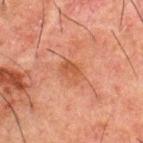Captured during whole-body skin photography for melanoma surveillance; the lesion was not biopsied. The subject is a male aged approximately 50. This is a cross-polarized tile. Measured at roughly 3 mm in maximum diameter. From the back. The total-body-photography lesion software estimated a classifier nevus-likeness of about 0/100 and a lesion-detection confidence of about 100/100. A roughly 15 mm field-of-view crop from a total-body skin photograph.